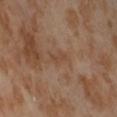| field | value |
|---|---|
| biopsy status | no biopsy performed (imaged during a skin exam) |
| size | about 2.5 mm |
| image | 15 mm crop, total-body photography |
| patient | female, aged approximately 55 |
| anatomic site | the right thigh |